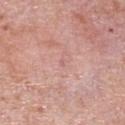Q: What kind of image is this?
A: ~15 mm tile from a whole-body skin photo
Q: Where on the body is the lesion?
A: the right upper arm
Q: Who is the patient?
A: male, aged around 75
Q: What lighting was used for the tile?
A: white-light illumination